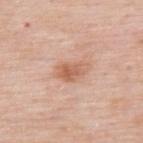Clinical impression: No biopsy was performed on this lesion — it was imaged during a full skin examination and was not determined to be concerning. Background: A 15 mm close-up tile from a total-body photography series done for melanoma screening. The patient is a male in their mid- to late 50s. An algorithmic analysis of the crop reported an area of roughly 7 mm², an eccentricity of roughly 0.8, and a symmetry-axis asymmetry near 0.35. The software also gave border irregularity of about 3 on a 0–10 scale, a color-variation rating of about 4.5/10, and radial color variation of about 1.5. From the upper back.